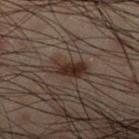Notes:
– subject — male, roughly 50 years of age
– body site — the right lower leg
– acquisition — ~15 mm tile from a whole-body skin photo